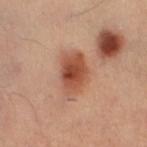workup: total-body-photography surveillance lesion; no biopsy | anatomic site: the leg | acquisition: ~15 mm crop, total-body skin-cancer survey | subject: female, in their mid- to late 30s | illumination: cross-polarized illumination | lesion size: ~4.5 mm (longest diameter).